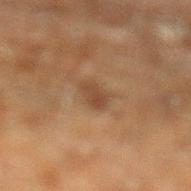biopsy_status: not biopsied; imaged during a skin examination
site: leg
image:
  source: total-body photography crop
  field_of_view_mm: 15
lesion_size:
  long_diameter_mm_approx: 2.5
patient:
  sex: male
  age_approx: 60